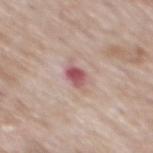<tbp_lesion>
  <site>mid back</site>
  <automated_metrics>
    <eccentricity>0.7</eccentricity>
    <shape_asymmetry>0.25</shape_asymmetry>
    <cielab_L>54</cielab_L>
    <cielab_a>27</cielab_a>
    <cielab_b>20</cielab_b>
    <vs_skin_darker_L>14.0</vs_skin_darker_L>
    <vs_skin_contrast_norm>9.5</vs_skin_contrast_norm>
    <nevus_likeness_0_100>0</nevus_likeness_0_100>
    <lesion_detection_confidence_0_100>100</lesion_detection_confidence_0_100>
  </automated_metrics>
  <image>
    <source>total-body photography crop</source>
    <field_of_view_mm>15</field_of_view_mm>
  </image>
  <lesion_size>
    <long_diameter_mm_approx>2.5</long_diameter_mm_approx>
  </lesion_size>
  <patient>
    <sex>male</sex>
    <age_approx>65</age_approx>
  </patient>
  <lighting>white-light</lighting>
</tbp_lesion>